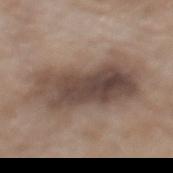Q: Was this lesion biopsied?
A: total-body-photography surveillance lesion; no biopsy
Q: Lesion size?
A: about 10.5 mm
Q: Illumination type?
A: white-light
Q: What did automated image analysis measure?
A: an area of roughly 37 mm² and a shape eccentricity near 0.9; a lesion-to-skin contrast of about 9.5 (normalized; higher = more distinct)
Q: Lesion location?
A: the mid back
Q: Who is the patient?
A: female, in their mid-70s
Q: What is the imaging modality?
A: ~15 mm crop, total-body skin-cancer survey